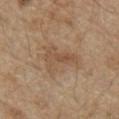Notes:
- follow-up · catalogued during a skin exam; not biopsied
- illumination · white-light
- image source · ~15 mm tile from a whole-body skin photo
- anatomic site · the front of the torso
- patient · male, aged around 70
- diameter · ~4.5 mm (longest diameter)